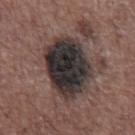workup = total-body-photography surveillance lesion; no biopsy
lesion size = ≈7.5 mm
automated metrics = a mean CIELAB color near L≈30 a*≈9 b*≈12 and about 17 CIELAB-L* units darker than the surrounding skin
image = total-body-photography crop, ~15 mm field of view
tile lighting = white-light
subject = male, about 70 years old
location = the abdomen